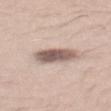Imaged during a routine full-body skin examination; the lesion was not biopsied and no histopathology is available. On the mid back. A 15 mm close-up extracted from a 3D total-body photography capture. A male patient, aged around 25. Imaged with white-light lighting. About 4.5 mm across.